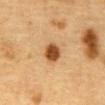The lesion was photographed on a routine skin check and not biopsied; there is no pathology result. A male subject, aged 83 or over. A 15 mm crop from a total-body photograph taken for skin-cancer surveillance. Longest diameter approximately 2.5 mm. The lesion is located on the abdomen.